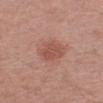| field | value |
|---|---|
| notes | total-body-photography surveillance lesion; no biopsy |
| lesion diameter | about 3 mm |
| subject | male, aged 48–52 |
| image | 15 mm crop, total-body photography |
| location | the arm |
| automated metrics | a lesion–skin lightness drop of about 8 and a normalized lesion–skin contrast near 6; a border-irregularity index near 2/10, a color-variation rating of about 2/10, and peripheral color asymmetry of about 1 |